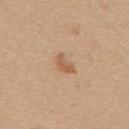Case summary:
– acquisition · ~15 mm tile from a whole-body skin photo
– size · ~3 mm (longest diameter)
– subject · female, aged 38 to 42
– site · the back
– image-analysis metrics · a lesion area of about 3 mm², a shape eccentricity near 0.85, and two-axis asymmetry of about 0.45; a lesion color around L≈58 a*≈19 b*≈35 in CIELAB and a normalized lesion–skin contrast near 7; internal color variation of about 1.5 on a 0–10 scale and radial color variation of about 0.5; a classifier nevus-likeness of about 70/100
– tile lighting · white-light illumination